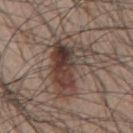Case summary:
* follow-up: no biopsy performed (imaged during a skin exam)
* lighting: white-light
* patient: male, about 45 years old
* anatomic site: the mid back
* acquisition: ~15 mm crop, total-body skin-cancer survey